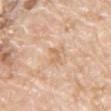Q: Was a biopsy performed?
A: no biopsy performed (imaged during a skin exam)
Q: What are the patient's age and sex?
A: male, roughly 60 years of age
Q: Lesion location?
A: the chest
Q: Lesion size?
A: about 3 mm
Q: Illumination type?
A: white-light
Q: What is the imaging modality?
A: ~15 mm tile from a whole-body skin photo